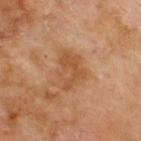follow-up: no biopsy performed (imaged during a skin exam) | patient: male, aged 68–72 | tile lighting: cross-polarized illumination | body site: the upper back | diameter: ≈4 mm | image-analysis metrics: a mean CIELAB color near L≈51 a*≈22 b*≈36, about 8 CIELAB-L* units darker than the surrounding skin, and a lesion-to-skin contrast of about 6 (normalized; higher = more distinct); border irregularity of about 3.5 on a 0–10 scale, a color-variation rating of about 4/10, and peripheral color asymmetry of about 1; a nevus-likeness score of about 0/100 and a lesion-detection confidence of about 100/100 | image source: 15 mm crop, total-body photography.